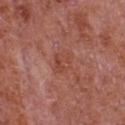notes — total-body-photography surveillance lesion; no biopsy | location — the chest | size — ≈3 mm | patient — male, aged around 65 | imaging modality — total-body-photography crop, ~15 mm field of view | automated lesion analysis — border irregularity of about 2 on a 0–10 scale, internal color variation of about 3 on a 0–10 scale, and radial color variation of about 1.5; a nevus-likeness score of about 0/100 and a lesion-detection confidence of about 100/100.